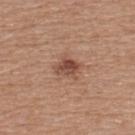Q: Is there a histopathology result?
A: no biopsy performed (imaged during a skin exam)